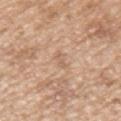{
  "biopsy_status": "not biopsied; imaged during a skin examination",
  "site": "left upper arm",
  "patient": {
    "sex": "male",
    "age_approx": 65
  },
  "image": {
    "source": "total-body photography crop",
    "field_of_view_mm": 15
  }
}